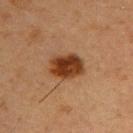Assessment: Captured during whole-body skin photography for melanoma surveillance; the lesion was not biopsied. Context: The tile uses cross-polarized illumination. A 15 mm crop from a total-body photograph taken for skin-cancer surveillance. The lesion is on the chest. The patient is a male aged approximately 55.